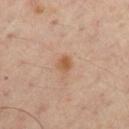workup: imaged on a skin check; not biopsied
acquisition: 15 mm crop, total-body photography
patient: male, about 45 years old
body site: the right upper arm
illumination: cross-polarized
lesion diameter: about 2 mm